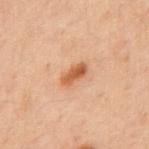A male patient, aged 58 to 62. The lesion is located on the front of the torso. This image is a 15 mm lesion crop taken from a total-body photograph.